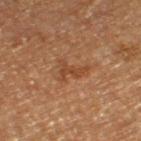biopsy status: no biopsy performed (imaged during a skin exam) | image source: ~15 mm crop, total-body skin-cancer survey | patient: male, aged 73 to 77 | anatomic site: the left thigh | illumination: cross-polarized.